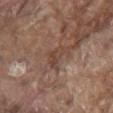The lesion was tiled from a total-body skin photograph and was not biopsied. The tile uses white-light illumination. The recorded lesion diameter is about 3.5 mm. On the mid back. A roughly 15 mm field-of-view crop from a total-body skin photograph. A male patient, approximately 80 years of age.